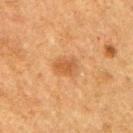Clinical impression: Part of a total-body skin-imaging series; this lesion was reviewed on a skin check and was not flagged for biopsy. Background: Longest diameter approximately 3 mm. Imaged with cross-polarized lighting. A 15 mm close-up extracted from a 3D total-body photography capture. The patient is a male about 75 years old. The lesion is on the right upper arm.